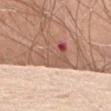Impression: Part of a total-body skin-imaging series; this lesion was reviewed on a skin check and was not flagged for biopsy. Clinical summary: A female patient, in their 70s. A 15 mm close-up tile from a total-body photography series done for melanoma screening. This is a white-light tile. From the abdomen.